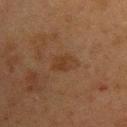Captured during whole-body skin photography for melanoma surveillance; the lesion was not biopsied. The tile uses cross-polarized illumination. A 15 mm close-up tile from a total-body photography series done for melanoma screening. Approximately 3.5 mm at its widest. The lesion is located on the upper back. The patient is a female aged 53 to 57.